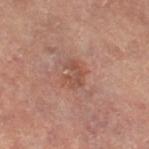<lesion>
<site>leg</site>
<lesion_size>
  <long_diameter_mm_approx>3.0</long_diameter_mm_approx>
</lesion_size>
<patient>
  <sex>female</sex>
  <age_approx>60</age_approx>
</patient>
<image>
  <source>total-body photography crop</source>
  <field_of_view_mm>15</field_of_view_mm>
</image>
</lesion>